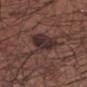notes — imaged on a skin check; not biopsied | tile lighting — white-light illumination | size — ~6 mm (longest diameter) | anatomic site — the left forearm | TBP lesion metrics — a lesion area of about 12 mm², an outline eccentricity of about 0.8 (0 = round, 1 = elongated), and a symmetry-axis asymmetry near 0.35; border irregularity of about 4 on a 0–10 scale and a peripheral color-asymmetry measure near 1.5 | subject — male, in their mid-50s | image — ~15 mm crop, total-body skin-cancer survey.